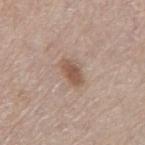Q: What is the imaging modality?
A: ~15 mm tile from a whole-body skin photo
Q: Automated lesion metrics?
A: an average lesion color of about L≈54 a*≈18 b*≈27 (CIELAB) and a lesion-to-skin contrast of about 8 (normalized; higher = more distinct)
Q: Who is the patient?
A: male, aged around 80
Q: What is the anatomic site?
A: the mid back